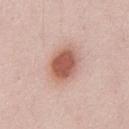No biopsy was performed on this lesion — it was imaged during a full skin examination and was not determined to be concerning.
The lesion is on the front of the torso.
A male patient, approximately 35 years of age.
Longest diameter approximately 4.5 mm.
Cropped from a whole-body photographic skin survey; the tile spans about 15 mm.